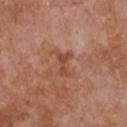location: the chest | lighting: white-light | acquisition: total-body-photography crop, ~15 mm field of view | subject: female, about 75 years old | lesion diameter: about 3 mm.